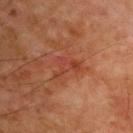The lesion is on the chest. This is a cross-polarized tile. The subject is a male approximately 55 years of age. This image is a 15 mm lesion crop taken from a total-body photograph.